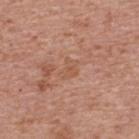– workup · total-body-photography surveillance lesion; no biopsy
– image source · total-body-photography crop, ~15 mm field of view
– automated metrics · an area of roughly 3.5 mm² and a shape eccentricity near 0.75; a normalized lesion–skin contrast near 5; a border-irregularity rating of about 4/10, a color-variation rating of about 2/10, and radial color variation of about 0.5; an automated nevus-likeness rating near 0 out of 100
– patient · male, aged around 70
– location · the upper back